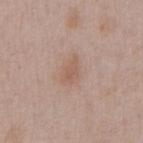Impression:
Part of a total-body skin-imaging series; this lesion was reviewed on a skin check and was not flagged for biopsy.
Acquisition and patient details:
The total-body-photography lesion software estimated a footprint of about 3.5 mm² and a shape eccentricity near 0.85. The software also gave an automated nevus-likeness rating near 15 out of 100. The subject is a male approximately 50 years of age. This is a white-light tile. Cropped from a whole-body photographic skin survey; the tile spans about 15 mm. The lesion is located on the chest.